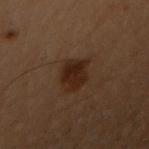| field | value |
|---|---|
| follow-up | imaged on a skin check; not biopsied |
| site | the right upper arm |
| lighting | cross-polarized |
| automated metrics | an area of roughly 9 mm², a shape eccentricity near 0.7, and a shape-asymmetry score of about 0.25 (0 = symmetric); a classifier nevus-likeness of about 85/100 and a detector confidence of about 100 out of 100 that the crop contains a lesion |
| acquisition | ~15 mm crop, total-body skin-cancer survey |
| subject | female, in their 50s |